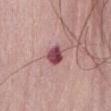Clinical summary:
On the front of the torso. Longest diameter approximately 2.5 mm. This is a white-light tile. A lesion tile, about 15 mm wide, cut from a 3D total-body photograph. A female subject, in their mid-60s.